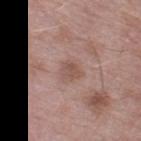Notes:
– notes · total-body-photography surveillance lesion; no biopsy
– patient · male, in their mid- to late 50s
– image-analysis metrics · an average lesion color of about L≈52 a*≈19 b*≈24 (CIELAB), a lesion–skin lightness drop of about 8, and a lesion-to-skin contrast of about 6 (normalized; higher = more distinct)
– lesion size · ~2.5 mm (longest diameter)
– lighting · white-light illumination
– image · ~15 mm tile from a whole-body skin photo
– body site · the left thigh